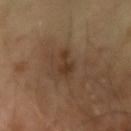Impression:
Part of a total-body skin-imaging series; this lesion was reviewed on a skin check and was not flagged for biopsy.
Background:
Imaged with cross-polarized lighting. A male subject approximately 65 years of age. Longest diameter approximately 4 mm. A 15 mm close-up extracted from a 3D total-body photography capture. An algorithmic analysis of the crop reported a footprint of about 4.5 mm², a shape eccentricity near 0.9, and two-axis asymmetry of about 0.5. And it measured a mean CIELAB color near L≈33 a*≈16 b*≈27, about 7 CIELAB-L* units darker than the surrounding skin, and a lesion-to-skin contrast of about 7 (normalized; higher = more distinct). The software also gave a peripheral color-asymmetry measure near 0.5.